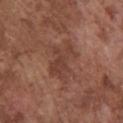<case>
<biopsy_status>not biopsied; imaged during a skin examination</biopsy_status>
<site>chest</site>
<image>
  <source>total-body photography crop</source>
  <field_of_view_mm>15</field_of_view_mm>
</image>
<patient>
  <sex>male</sex>
  <age_approx>75</age_approx>
</patient>
</case>